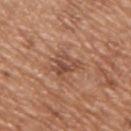| key | value |
|---|---|
| notes | no biopsy performed (imaged during a skin exam) |
| body site | the upper back |
| imaging modality | ~15 mm tile from a whole-body skin photo |
| subject | male, in their mid- to late 60s |
| diameter | ≈3.5 mm |
| TBP lesion metrics | a mean CIELAB color near L≈49 a*≈22 b*≈30 and about 9 CIELAB-L* units darker than the surrounding skin; a color-variation rating of about 5.5/10 and peripheral color asymmetry of about 2 |